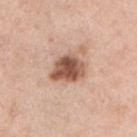Clinical impression: Captured during whole-body skin photography for melanoma surveillance; the lesion was not biopsied. Image and clinical context: About 4 mm across. Automated tile analysis of the lesion measured a lesion area of about 11 mm², an outline eccentricity of about 0.6 (0 = round, 1 = elongated), and a symmetry-axis asymmetry near 0.2. The analysis additionally found a mean CIELAB color near L≈55 a*≈21 b*≈30, roughly 17 lightness units darker than nearby skin, and a lesion-to-skin contrast of about 11 (normalized; higher = more distinct). And it measured a nevus-likeness score of about 70/100 and a lesion-detection confidence of about 100/100. This is a white-light tile. From the left upper arm. A roughly 15 mm field-of-view crop from a total-body skin photograph. The patient is a male about 40 years old.